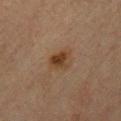follow-up — catalogued during a skin exam; not biopsied | tile lighting — cross-polarized | location — the chest | size — ≈3 mm | image source — 15 mm crop, total-body photography | patient — male, about 60 years old.